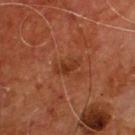* follow-up — imaged on a skin check; not biopsied
* automated metrics — an average lesion color of about L≈32 a*≈25 b*≈32 (CIELAB), roughly 7 lightness units darker than nearby skin, and a normalized border contrast of about 6.5; an automated nevus-likeness rating near 0 out of 100 and lesion-presence confidence of about 100/100
* lesion size — about 3 mm
* anatomic site — the chest
* acquisition — ~15 mm crop, total-body skin-cancer survey
* subject — male, approximately 55 years of age